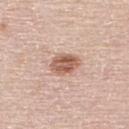Q: Was a biopsy performed?
A: catalogued during a skin exam; not biopsied
Q: What kind of image is this?
A: ~15 mm tile from a whole-body skin photo
Q: Where on the body is the lesion?
A: the upper back
Q: What lighting was used for the tile?
A: white-light illumination
Q: Patient demographics?
A: male, aged 58 to 62